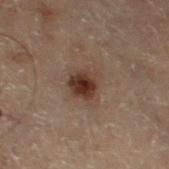The lesion was tiled from a total-body skin photograph and was not biopsied. A male patient aged 68–72. From the left thigh. Cropped from a total-body skin-imaging series; the visible field is about 15 mm. Longest diameter approximately 4 mm. The tile uses cross-polarized illumination.